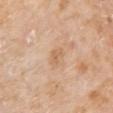<case>
  <biopsy_status>not biopsied; imaged during a skin examination</biopsy_status>
  <image>
    <source>total-body photography crop</source>
    <field_of_view_mm>15</field_of_view_mm>
  </image>
  <lesion_size>
    <long_diameter_mm_approx>2.5</long_diameter_mm_approx>
  </lesion_size>
  <patient>
    <sex>male</sex>
    <age_approx>75</age_approx>
  </patient>
  <site>arm</site>
  <automated_metrics>
    <nevus_likeness_0_100>0</nevus_likeness_0_100>
    <lesion_detection_confidence_0_100>100</lesion_detection_confidence_0_100>
  </automated_metrics>
</case>